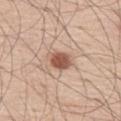Imaged during a routine full-body skin examination; the lesion was not biopsied and no histopathology is available. Cropped from a total-body skin-imaging series; the visible field is about 15 mm. From the left thigh. An algorithmic analysis of the crop reported an area of roughly 6 mm² and a symmetry-axis asymmetry near 0.2. The software also gave an average lesion color of about L≈55 a*≈22 b*≈28 (CIELAB) and about 14 CIELAB-L* units darker than the surrounding skin. The patient is a male aged approximately 60.